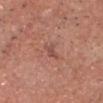This lesion was catalogued during total-body skin photography and was not selected for biopsy. The subject is a male aged 58–62. From the head or neck. The lesion's longest dimension is about 2.5 mm. A roughly 15 mm field-of-view crop from a total-body skin photograph. This is a white-light tile.